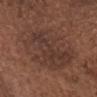Notes:
– follow-up — catalogued during a skin exam; not biopsied
– imaging modality — 15 mm crop, total-body photography
– anatomic site — the chest
– automated metrics — a lesion color around L≈37 a*≈18 b*≈23 in CIELAB; a lesion-detection confidence of about 100/100
– tile lighting — white-light illumination
– patient — male, approximately 75 years of age